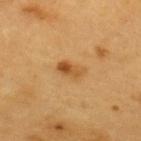Imaged during a routine full-body skin examination; the lesion was not biopsied and no histopathology is available. A lesion tile, about 15 mm wide, cut from a 3D total-body photograph. The lesion's longest dimension is about 3 mm. On the upper back. The subject is a male aged 58 to 62. Imaged with cross-polarized lighting.